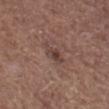No biopsy was performed on this lesion — it was imaged during a full skin examination and was not determined to be concerning.
The lesion is located on the abdomen.
A male patient roughly 70 years of age.
Longest diameter approximately 3.5 mm.
A 15 mm close-up extracted from a 3D total-body photography capture.
An algorithmic analysis of the crop reported an average lesion color of about L≈41 a*≈18 b*≈21 (CIELAB), roughly 8 lightness units darker than nearby skin, and a lesion-to-skin contrast of about 6.5 (normalized; higher = more distinct). The software also gave a classifier nevus-likeness of about 40/100 and a detector confidence of about 100 out of 100 that the crop contains a lesion.
This is a white-light tile.